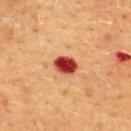anatomic site = the upper back | patient = male, approximately 60 years of age | diameter = ≈3 mm | lighting = cross-polarized illumination | image source = total-body-photography crop, ~15 mm field of view | image-analysis metrics = an area of roughly 6 mm² and two-axis asymmetry of about 0.2; a border-irregularity rating of about 1.5/10, a within-lesion color-variation index near 4.5/10, and a peripheral color-asymmetry measure near 1; an automated nevus-likeness rating near 0 out of 100 and a lesion-detection confidence of about 100/100.